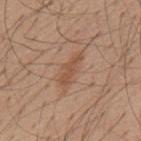Q: Was this lesion biopsied?
A: no biopsy performed (imaged during a skin exam)
Q: Patient demographics?
A: male, approximately 55 years of age
Q: What kind of image is this?
A: 15 mm crop, total-body photography
Q: What lighting was used for the tile?
A: white-light
Q: What did automated image analysis measure?
A: a footprint of about 5.5 mm² and a shape eccentricity near 0.95; a lesion color around L≈52 a*≈20 b*≈31 in CIELAB, roughly 8 lightness units darker than nearby skin, and a normalized lesion–skin contrast near 6; a peripheral color-asymmetry measure near 0.5
Q: Where on the body is the lesion?
A: the mid back
Q: How large is the lesion?
A: ≈4.5 mm